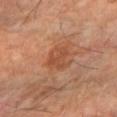The lesion was tiled from a total-body skin photograph and was not biopsied. The subject is a male aged approximately 70. Cropped from a total-body skin-imaging series; the visible field is about 15 mm. Located on the right forearm.